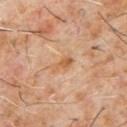{"biopsy_status": "not biopsied; imaged during a skin examination", "lighting": "cross-polarized", "lesion_size": {"long_diameter_mm_approx": 2.5}, "patient": {"sex": "male", "age_approx": 60}, "automated_metrics": {"area_mm2_approx": 3.0, "eccentricity": 0.85, "shape_asymmetry": 0.35, "nevus_likeness_0_100": 0, "lesion_detection_confidence_0_100": 100}, "site": "chest", "image": {"source": "total-body photography crop", "field_of_view_mm": 15}}